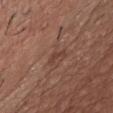Captured during whole-body skin photography for melanoma surveillance; the lesion was not biopsied.
The recorded lesion diameter is about 3 mm.
Automated image analysis of the tile measured a lesion area of about 2.5 mm² and a shape eccentricity near 0.9. The analysis additionally found an average lesion color of about L≈41 a*≈20 b*≈26 (CIELAB), roughly 7 lightness units darker than nearby skin, and a normalized lesion–skin contrast near 5.5. It also reported a border-irregularity rating of about 5.5/10 and a peripheral color-asymmetry measure near 0.
Captured under white-light illumination.
A 15 mm close-up tile from a total-body photography series done for melanoma screening.
The lesion is on the chest.
The subject is a male in their mid- to late 70s.